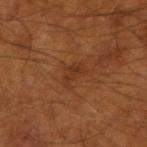Imaged during a routine full-body skin examination; the lesion was not biopsied and no histopathology is available. A 15 mm crop from a total-body photograph taken for skin-cancer surveillance. A male patient, in their mid-50s. Imaged with cross-polarized lighting. The lesion is located on the right forearm. The lesion-visualizer software estimated a footprint of about 5 mm². It also reported a lesion color around L≈33 a*≈23 b*≈32 in CIELAB, a lesion–skin lightness drop of about 6, and a normalized lesion–skin contrast near 5.5. The software also gave a border-irregularity rating of about 5.5/10 and radial color variation of about 0.5. And it measured a lesion-detection confidence of about 100/100.